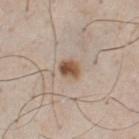Impression: This lesion was catalogued during total-body skin photography and was not selected for biopsy. Context: From the chest. The patient is a male approximately 50 years of age. A roughly 15 mm field-of-view crop from a total-body skin photograph. The total-body-photography lesion software estimated an area of roughly 4.5 mm², an eccentricity of roughly 0.55, and two-axis asymmetry of about 0.2. And it measured a border-irregularity rating of about 1.5/10, a within-lesion color-variation index near 5/10, and radial color variation of about 2. Measured at roughly 2.5 mm in maximum diameter. The tile uses white-light illumination.